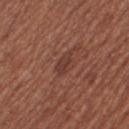follow-up: no biopsy performed (imaged during a skin exam) | lesion diameter: ≈3 mm | body site: the right thigh | patient: female, aged around 55 | automated metrics: an area of roughly 4 mm², an outline eccentricity of about 0.8 (0 = round, 1 = elongated), and a symmetry-axis asymmetry near 0.25; a mean CIELAB color near L≈38 a*≈23 b*≈25 and a lesion–skin lightness drop of about 7 | image: 15 mm crop, total-body photography.